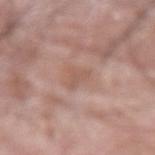workup: total-body-photography surveillance lesion; no biopsy
subject: male, in their mid- to late 80s or older
site: the right upper arm
diameter: about 2.5 mm
TBP lesion metrics: an area of roughly 3.5 mm², an outline eccentricity of about 0.65 (0 = round, 1 = elongated), and a symmetry-axis asymmetry near 0.45; a color-variation rating of about 1.5/10 and peripheral color asymmetry of about 0.5; an automated nevus-likeness rating near 0 out of 100 and a detector confidence of about 100 out of 100 that the crop contains a lesion
acquisition: total-body-photography crop, ~15 mm field of view